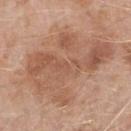Imaged during a routine full-body skin examination; the lesion was not biopsied and no histopathology is available.
The lesion is located on the right forearm.
A male patient, roughly 75 years of age.
A 15 mm close-up tile from a total-body photography series done for melanoma screening.
The tile uses white-light illumination.